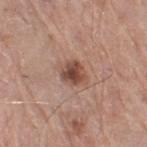Clinical impression: Imaged during a routine full-body skin examination; the lesion was not biopsied and no histopathology is available. Image and clinical context: A 15 mm close-up tile from a total-body photography series done for melanoma screening. From the leg. Automated tile analysis of the lesion measured a footprint of about 6 mm² and an outline eccentricity of about 0.5 (0 = round, 1 = elongated). The analysis additionally found a classifier nevus-likeness of about 90/100. The lesion's longest dimension is about 3 mm. A male patient aged 78–82.